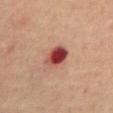| field | value |
|---|---|
| workup | total-body-photography surveillance lesion; no biopsy |
| patient | male, aged around 70 |
| location | the mid back |
| automated lesion analysis | a footprint of about 7 mm²; a lesion color around L≈43 a*≈31 b*≈26 in CIELAB, roughly 18 lightness units darker than nearby skin, and a lesion-to-skin contrast of about 13 (normalized; higher = more distinct) |
| illumination | cross-polarized illumination |
| acquisition | total-body-photography crop, ~15 mm field of view |